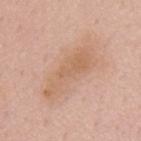Notes:
- biopsy status — no biopsy performed (imaged during a skin exam)
- image — total-body-photography crop, ~15 mm field of view
- subject — male, about 55 years old
- anatomic site — the mid back